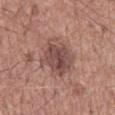The lesion was photographed on a routine skin check and not biopsied; there is no pathology result. The lesion is on the mid back. The subject is a male aged 53–57. This image is a 15 mm lesion crop taken from a total-body photograph.